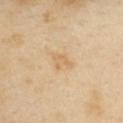The lesion was photographed on a routine skin check and not biopsied; there is no pathology result. Cropped from a total-body skin-imaging series; the visible field is about 15 mm. Automated image analysis of the tile measured a lesion area of about 4 mm² and an outline eccentricity of about 0.7 (0 = round, 1 = elongated). It also reported a border-irregularity index near 4/10, a color-variation rating of about 1/10, and peripheral color asymmetry of about 0.5. The analysis additionally found a nevus-likeness score of about 0/100 and a lesion-detection confidence of about 100/100. The subject is a female in their 40s. The lesion is located on the front of the torso. Captured under cross-polarized illumination. Approximately 2.5 mm at its widest.